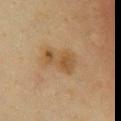Assessment:
The lesion was tiled from a total-body skin photograph and was not biopsied.
Acquisition and patient details:
The recorded lesion diameter is about 4.5 mm. This is a cross-polarized tile. From the chest. A female subject, aged 38–42. A 15 mm close-up extracted from a 3D total-body photography capture.